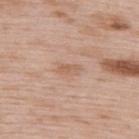<case>
<biopsy_status>not biopsied; imaged during a skin examination</biopsy_status>
<image>
  <source>total-body photography crop</source>
  <field_of_view_mm>15</field_of_view_mm>
</image>
<site>upper back</site>
<patient>
  <sex>male</sex>
  <age_approx>50</age_approx>
</patient>
<lesion_size>
  <long_diameter_mm_approx>3.0</long_diameter_mm_approx>
</lesion_size>
<lighting>white-light</lighting>
</case>